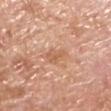| key | value |
|---|---|
| follow-up | no biopsy performed (imaged during a skin exam) |
| subject | male, aged 78–82 |
| image source | ~15 mm crop, total-body skin-cancer survey |
| body site | the left lower leg |